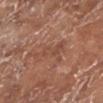{"biopsy_status": "not biopsied; imaged during a skin examination", "patient": {"sex": "female", "age_approx": 75}, "lighting": "white-light", "site": "left lower leg", "lesion_size": {"long_diameter_mm_approx": 5.0}, "image": {"source": "total-body photography crop", "field_of_view_mm": 15}}